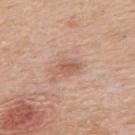A 15 mm close-up extracted from a 3D total-body photography capture. A male subject aged 48 to 52. The lesion is on the upper back.